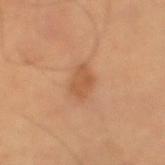Notes:
• biopsy status: imaged on a skin check; not biopsied
• acquisition: ~15 mm crop, total-body skin-cancer survey
• diameter: ≈3.5 mm
• subject: male, aged 48–52
• lighting: cross-polarized illumination
• location: the arm